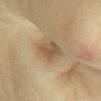imaging modality — total-body-photography crop, ~15 mm field of view | image-analysis metrics — a within-lesion color-variation index near 3/10 and peripheral color asymmetry of about 1 | site — the right lower leg | illumination — cross-polarized illumination | lesion diameter — about 3 mm | patient — female, aged 53–57.